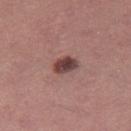{"biopsy_status": "not biopsied; imaged during a skin examination", "lighting": "white-light", "patient": {"sex": "male", "age_approx": 40}, "automated_metrics": {"area_mm2_approx": 5.0, "eccentricity": 0.8, "shape_asymmetry": 0.2, "cielab_L": 42, "cielab_a": 21, "cielab_b": 20, "vs_skin_darker_L": 15.0, "nevus_likeness_0_100": 85, "lesion_detection_confidence_0_100": 100}, "lesion_size": {"long_diameter_mm_approx": 3.0}, "site": "leg", "image": {"source": "total-body photography crop", "field_of_view_mm": 15}}